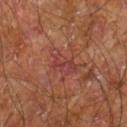Clinical impression:
Captured during whole-body skin photography for melanoma surveillance; the lesion was not biopsied.
Background:
The subject is a male aged approximately 60. About 4 mm across. Imaged with cross-polarized lighting. Located on the right leg. Automated image analysis of the tile measured an eccentricity of roughly 0.85 and two-axis asymmetry of about 0.4. The analysis additionally found an automated nevus-likeness rating near 0 out of 100. A roughly 15 mm field-of-view crop from a total-body skin photograph.